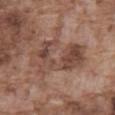This lesion was catalogued during total-body skin photography and was not selected for biopsy.
A male patient in their mid- to late 70s.
Cropped from a total-body skin-imaging series; the visible field is about 15 mm.
The lesion's longest dimension is about 7.5 mm.
The total-body-photography lesion software estimated an average lesion color of about L≈46 a*≈19 b*≈26 (CIELAB), a lesion–skin lightness drop of about 9, and a normalized lesion–skin contrast near 7.
The tile uses white-light illumination.
The lesion is on the abdomen.